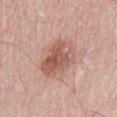Captured under white-light illumination. Located on the lower back. The recorded lesion diameter is about 5.5 mm. A region of skin cropped from a whole-body photographic capture, roughly 15 mm wide. A male patient, aged approximately 50.